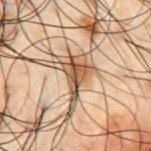Impression: This lesion was catalogued during total-body skin photography and was not selected for biopsy. Clinical summary: Captured under cross-polarized illumination. A roughly 15 mm field-of-view crop from a total-body skin photograph. Measured at roughly 4.5 mm in maximum diameter. A male patient in their 50s. The total-body-photography lesion software estimated an area of roughly 6.5 mm² and a shape eccentricity near 0.85. And it measured a lesion color around L≈40 a*≈14 b*≈27 in CIELAB and a lesion-to-skin contrast of about 11.5 (normalized; higher = more distinct). And it measured a border-irregularity rating of about 5.5/10, a color-variation rating of about 5.5/10, and peripheral color asymmetry of about 2. From the chest.